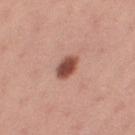Recorded during total-body skin imaging; not selected for excision or biopsy.